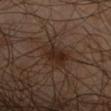Background: The tile uses cross-polarized illumination. Automated tile analysis of the lesion measured an area of roughly 10 mm² and a shape eccentricity near 0.7. About 4.5 mm across. The lesion is located on the right upper arm. This image is a 15 mm lesion crop taken from a total-body photograph. A male subject aged approximately 65.